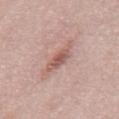<tbp_lesion>
<biopsy_status>not biopsied; imaged during a skin examination</biopsy_status>
<patient>
  <sex>male</sex>
  <age_approx>55</age_approx>
</patient>
<site>mid back</site>
<image>
  <source>total-body photography crop</source>
  <field_of_view_mm>15</field_of_view_mm>
</image>
<lesion_size>
  <long_diameter_mm_approx>4.0</long_diameter_mm_approx>
</lesion_size>
<lighting>white-light</lighting>
</tbp_lesion>